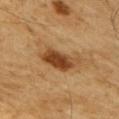Part of a total-body skin-imaging series; this lesion was reviewed on a skin check and was not flagged for biopsy. The patient is a male about 60 years old. Located on the right upper arm. A lesion tile, about 15 mm wide, cut from a 3D total-body photograph.